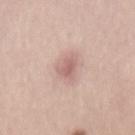Q: Is there a histopathology result?
A: no biopsy performed (imaged during a skin exam)
Q: What lighting was used for the tile?
A: white-light
Q: Patient demographics?
A: male, aged 28–32
Q: What kind of image is this?
A: ~15 mm crop, total-body skin-cancer survey
Q: Lesion size?
A: about 3.5 mm
Q: What did automated image analysis measure?
A: a lesion area of about 6 mm² and a symmetry-axis asymmetry near 0.3; a detector confidence of about 100 out of 100 that the crop contains a lesion